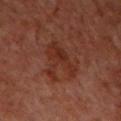Q: Is there a histopathology result?
A: no biopsy performed (imaged during a skin exam)
Q: What is the anatomic site?
A: the back
Q: What is the imaging modality?
A: ~15 mm crop, total-body skin-cancer survey
Q: Automated lesion metrics?
A: a lesion area of about 12 mm², an eccentricity of roughly 0.7, and two-axis asymmetry of about 0.6; a border-irregularity rating of about 9/10 and internal color variation of about 3 on a 0–10 scale; an automated nevus-likeness rating near 0 out of 100
Q: How was the tile lit?
A: cross-polarized
Q: Patient demographics?
A: male, aged approximately 60